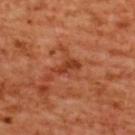This lesion was catalogued during total-body skin photography and was not selected for biopsy. The subject is a female aged 53 to 57. On the upper back. Cropped from a whole-body photographic skin survey; the tile spans about 15 mm.